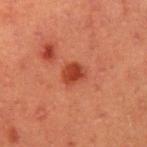notes: catalogued during a skin exam; not biopsied
image source: total-body-photography crop, ~15 mm field of view
lighting: cross-polarized
patient: male, about 40 years old
site: the right upper arm